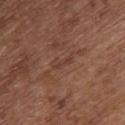This lesion was catalogued during total-body skin photography and was not selected for biopsy.
The tile uses white-light illumination.
A 15 mm close-up tile from a total-body photography series done for melanoma screening.
From the upper back.
The subject is a female aged approximately 80.